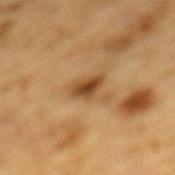No biopsy was performed on this lesion — it was imaged during a full skin examination and was not determined to be concerning. The tile uses cross-polarized illumination. A male patient, aged around 85 or older. Approximately 3 mm at its widest. A 15 mm close-up tile from a total-body photography series done for melanoma screening. The lesion is on the mid back.